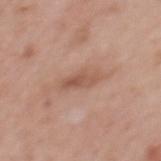notes = no biopsy performed (imaged during a skin exam)
imaging modality = 15 mm crop, total-body photography
subject = female, roughly 50 years of age
site = the back